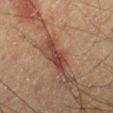imaging modality: ~15 mm crop, total-body skin-cancer survey; body site: the left lower leg; subject: male, about 60 years old.